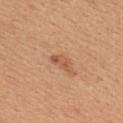| field | value |
|---|---|
| follow-up | imaged on a skin check; not biopsied |
| size | ~2.5 mm (longest diameter) |
| subject | female, about 30 years old |
| site | the upper back |
| illumination | white-light illumination |
| image | ~15 mm crop, total-body skin-cancer survey |